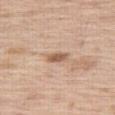Q: Was a biopsy performed?
A: catalogued during a skin exam; not biopsied
Q: What did automated image analysis measure?
A: an average lesion color of about L≈59 a*≈18 b*≈31 (CIELAB), about 12 CIELAB-L* units darker than the surrounding skin, and a normalized border contrast of about 7.5; a classifier nevus-likeness of about 55/100
Q: Lesion location?
A: the leg
Q: What are the patient's age and sex?
A: male, aged 68–72
Q: How was the tile lit?
A: white-light
Q: How large is the lesion?
A: ~3 mm (longest diameter)
Q: What kind of image is this?
A: 15 mm crop, total-body photography